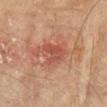- follow-up — imaged on a skin check; not biopsied
- body site — the left upper arm
- illumination — cross-polarized
- patient — male, approximately 75 years of age
- image — ~15 mm crop, total-body skin-cancer survey